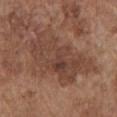biopsy status: catalogued during a skin exam; not biopsied | patient: male, roughly 75 years of age | site: the front of the torso | size: ≈9.5 mm | acquisition: 15 mm crop, total-body photography.